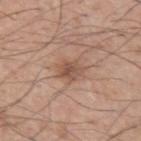<lesion>
<biopsy_status>not biopsied; imaged during a skin examination</biopsy_status>
<patient>
  <sex>male</sex>
  <age_approx>55</age_approx>
</patient>
<automated_metrics>
  <area_mm2_approx>5.5</area_mm2_approx>
  <eccentricity>0.55</eccentricity>
  <shape_asymmetry>0.25</shape_asymmetry>
  <cielab_L>52</cielab_L>
  <cielab_a>19</cielab_a>
  <cielab_b>28</cielab_b>
  <vs_skin_contrast_norm>7.0</vs_skin_contrast_norm>
  <color_variation_0_10>4.0</color_variation_0_10>
  <peripheral_color_asymmetry>1.5</peripheral_color_asymmetry>
  <nevus_likeness_0_100>30</nevus_likeness_0_100>
  <lesion_detection_confidence_0_100>100</lesion_detection_confidence_0_100>
</automated_metrics>
<lighting>white-light</lighting>
<lesion_size>
  <long_diameter_mm_approx>3.0</long_diameter_mm_approx>
</lesion_size>
<image>
  <source>total-body photography crop</source>
  <field_of_view_mm>15</field_of_view_mm>
</image>
</lesion>